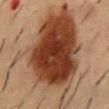Impression:
Part of a total-body skin-imaging series; this lesion was reviewed on a skin check and was not flagged for biopsy.
Background:
Captured under cross-polarized illumination. The lesion is located on the back. Longest diameter approximately 15.5 mm. The subject is a male aged around 55. A region of skin cropped from a whole-body photographic capture, roughly 15 mm wide.